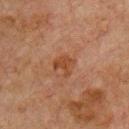Image and clinical context: The lesion-visualizer software estimated a border-irregularity index near 5/10, a color-variation rating of about 1/10, and a peripheral color-asymmetry measure near 0.5. The analysis additionally found a nevus-likeness score of about 0/100. A region of skin cropped from a whole-body photographic capture, roughly 15 mm wide. The tile uses cross-polarized illumination. The lesion is located on the chest. About 2.5 mm across. The subject is a male about 60 years old.